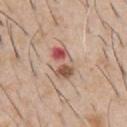Q: Was this lesion biopsied?
A: total-body-photography surveillance lesion; no biopsy
Q: What is the lesion's diameter?
A: ≈4.5 mm
Q: Lesion location?
A: the chest
Q: Who is the patient?
A: male, approximately 60 years of age
Q: What kind of image is this?
A: ~15 mm tile from a whole-body skin photo
Q: What did automated image analysis measure?
A: a lesion area of about 8.5 mm² and an eccentricity of roughly 0.85; an average lesion color of about L≈55 a*≈23 b*≈27 (CIELAB) and roughly 13 lightness units darker than nearby skin; border irregularity of about 3.5 on a 0–10 scale, a within-lesion color-variation index near 10/10, and peripheral color asymmetry of about 6; a nevus-likeness score of about 0/100 and a detector confidence of about 100 out of 100 that the crop contains a lesion
Q: How was the tile lit?
A: white-light